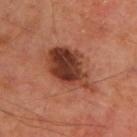Imaged during a routine full-body skin examination; the lesion was not biopsied and no histopathology is available.
Measured at roughly 7 mm in maximum diameter.
Captured under cross-polarized illumination.
A male patient approximately 65 years of age.
On the upper back.
An algorithmic analysis of the crop reported an outline eccentricity of about 0.8 (0 = round, 1 = elongated) and a shape-asymmetry score of about 0.2 (0 = symmetric). The software also gave an average lesion color of about L≈39 a*≈25 b*≈30 (CIELAB), roughly 14 lightness units darker than nearby skin, and a normalized lesion–skin contrast near 11. The analysis additionally found a border-irregularity rating of about 3/10, a within-lesion color-variation index near 8.5/10, and peripheral color asymmetry of about 3.
A close-up tile cropped from a whole-body skin photograph, about 15 mm across.